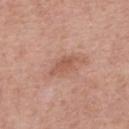notes: imaged on a skin check; not biopsied
image: ~15 mm crop, total-body skin-cancer survey
lesion size: ≈3.5 mm
site: the back
subject: male, roughly 65 years of age
TBP lesion metrics: an area of roughly 4 mm² and a shape-asymmetry score of about 0.25 (0 = symmetric); a classifier nevus-likeness of about 0/100 and a lesion-detection confidence of about 100/100
tile lighting: white-light illumination